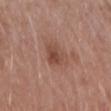This lesion was catalogued during total-body skin photography and was not selected for biopsy. The tile uses white-light illumination. The lesion is located on the arm. A female patient, aged around 50. A 15 mm close-up tile from a total-body photography series done for melanoma screening. The recorded lesion diameter is about 3.5 mm. Automated image analysis of the tile measured a footprint of about 7.5 mm², an outline eccentricity of about 0.75 (0 = round, 1 = elongated), and two-axis asymmetry of about 0.3. The software also gave a classifier nevus-likeness of about 55/100 and a lesion-detection confidence of about 100/100.